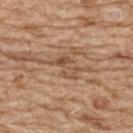notes: total-body-photography surveillance lesion; no biopsy | location: the upper back | subject: female, aged 68–72 | imaging modality: total-body-photography crop, ~15 mm field of view.